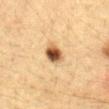The lesion was tiled from a total-body skin photograph and was not biopsied. Located on the abdomen. The total-body-photography lesion software estimated an automated nevus-likeness rating near 100 out of 100 and a lesion-detection confidence of about 100/100. A lesion tile, about 15 mm wide, cut from a 3D total-body photograph. A female subject, about 30 years old. Longest diameter approximately 3 mm.